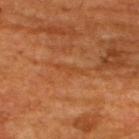No biopsy was performed on this lesion — it was imaged during a full skin examination and was not determined to be concerning.
The patient is a male in their 60s.
Cropped from a whole-body photographic skin survey; the tile spans about 15 mm.
From the upper back.